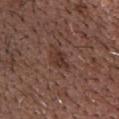The lesion was photographed on a routine skin check and not biopsied; there is no pathology result.
The lesion is on the head or neck.
This image is a 15 mm lesion crop taken from a total-body photograph.
A male subject roughly 65 years of age.